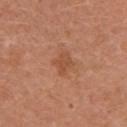<tbp_lesion>
  <lesion_size>
    <long_diameter_mm_approx>2.5</long_diameter_mm_approx>
  </lesion_size>
  <image>
    <source>total-body photography crop</source>
    <field_of_view_mm>15</field_of_view_mm>
  </image>
  <patient>
    <sex>female</sex>
    <age_approx>50</age_approx>
  </patient>
  <site>left upper arm</site>
  <automated_metrics>
    <area_mm2_approx>4.5</area_mm2_approx>
    <eccentricity>0.55</eccentricity>
    <shape_asymmetry>0.25</shape_asymmetry>
  </automated_metrics>
</tbp_lesion>